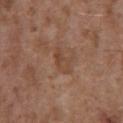Clinical impression:
Captured during whole-body skin photography for melanoma surveillance; the lesion was not biopsied.
Image and clinical context:
Longest diameter approximately 3 mm. From the abdomen. The total-body-photography lesion software estimated an average lesion color of about L≈44 a*≈19 b*≈29 (CIELAB), about 6 CIELAB-L* units darker than the surrounding skin, and a lesion-to-skin contrast of about 5.5 (normalized; higher = more distinct). Captured under white-light illumination. Cropped from a total-body skin-imaging series; the visible field is about 15 mm. A male subject about 75 years old.